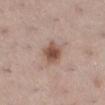The lesion was photographed on a routine skin check and not biopsied; there is no pathology result.
Located on the right lower leg.
An algorithmic analysis of the crop reported a mean CIELAB color near L≈51 a*≈20 b*≈26, about 13 CIELAB-L* units darker than the surrounding skin, and a normalized lesion–skin contrast near 9.5. It also reported a classifier nevus-likeness of about 95/100 and a lesion-detection confidence of about 100/100.
A 15 mm close-up tile from a total-body photography series done for melanoma screening.
This is a white-light tile.
The subject is a female in their 40s.
The lesion's longest dimension is about 3 mm.